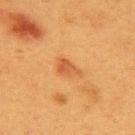The lesion was tiled from a total-body skin photograph and was not biopsied. This image is a 15 mm lesion crop taken from a total-body photograph. The patient is a female roughly 40 years of age. About 3 mm across. On the upper back.